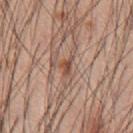Clinical impression: The lesion was tiled from a total-body skin photograph and was not biopsied. Context: The lesion is on the chest. A male patient in their 60s. Captured under white-light illumination. Measured at roughly 2.5 mm in maximum diameter. A region of skin cropped from a whole-body photographic capture, roughly 15 mm wide. The lesion-visualizer software estimated an average lesion color of about L≈50 a*≈20 b*≈29 (CIELAB), roughly 10 lightness units darker than nearby skin, and a lesion-to-skin contrast of about 7.5 (normalized; higher = more distinct). And it measured border irregularity of about 5 on a 0–10 scale and radial color variation of about 0.5.